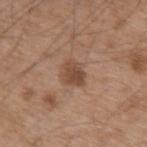This lesion was catalogued during total-body skin photography and was not selected for biopsy.